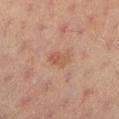Case summary:
* notes: imaged on a skin check; not biopsied
* patient: female, aged approximately 20
* imaging modality: total-body-photography crop, ~15 mm field of view
* site: the left lower leg
* size: ≈3 mm
* automated metrics: an average lesion color of about L≈47 a*≈19 b*≈27 (CIELAB); border irregularity of about 3.5 on a 0–10 scale, internal color variation of about 3.5 on a 0–10 scale, and radial color variation of about 1
* tile lighting: cross-polarized